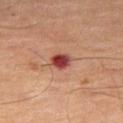Part of a total-body skin-imaging series; this lesion was reviewed on a skin check and was not flagged for biopsy.
Automated tile analysis of the lesion measured a lesion color around L≈40 a*≈30 b*≈26 in CIELAB and a lesion-to-skin contrast of about 12 (normalized; higher = more distinct).
The recorded lesion diameter is about 2.5 mm.
On the left thigh.
A close-up tile cropped from a whole-body skin photograph, about 15 mm across.
A male patient aged 63–67.
This is a cross-polarized tile.